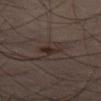Findings:
• biopsy status — catalogued during a skin exam; not biopsied
• acquisition — ~15 mm tile from a whole-body skin photo
• lighting — cross-polarized
• automated lesion analysis — an area of roughly 7.5 mm², a shape eccentricity near 0.95, and two-axis asymmetry of about 0.45; an average lesion color of about L≈28 a*≈11 b*≈17 (CIELAB), roughly 6 lightness units darker than nearby skin, and a lesion-to-skin contrast of about 6.5 (normalized; higher = more distinct); border irregularity of about 6.5 on a 0–10 scale, a color-variation rating of about 5/10, and radial color variation of about 1.5
• size — ~5.5 mm (longest diameter)
• patient — male, aged around 50
• body site — the lower back